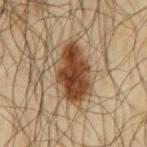Imaged during a routine full-body skin examination; the lesion was not biopsied and no histopathology is available.
The tile uses cross-polarized illumination.
Cropped from a whole-body photographic skin survey; the tile spans about 15 mm.
The lesion is located on the mid back.
About 7 mm across.
A male subject, about 65 years old.